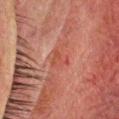workup — catalogued during a skin exam; not biopsied | body site — the head or neck | image source — 15 mm crop, total-body photography | diameter — ≈2.5 mm | illumination — cross-polarized illumination | patient — male, in their 60s.